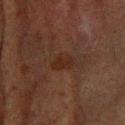lighting: cross-polarized
lesion_size:
  long_diameter_mm_approx: 3.0
patient:
  sex: male
  age_approx: 80
automated_metrics:
  border_irregularity_0_10: 2.5
  color_variation_0_10: 1.5
  peripheral_color_asymmetry: 0.5
  nevus_likeness_0_100: 20
  lesion_detection_confidence_0_100: 100
site: right forearm
image:
  source: total-body photography crop
  field_of_view_mm: 15